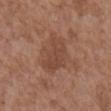This lesion was catalogued during total-body skin photography and was not selected for biopsy.
Imaged with white-light lighting.
Approximately 4.5 mm at its widest.
A roughly 15 mm field-of-view crop from a total-body skin photograph.
A male patient in their mid- to late 70s.
The lesion is on the abdomen.